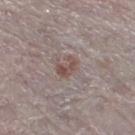The lesion was tiled from a total-body skin photograph and was not biopsied. The lesion's longest dimension is about 2.5 mm. A female subject, approximately 65 years of age. A roughly 15 mm field-of-view crop from a total-body skin photograph. From the left lower leg.